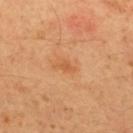The lesion was photographed on a routine skin check and not biopsied; there is no pathology result. Located on the upper back. A lesion tile, about 15 mm wide, cut from a 3D total-body photograph. A male patient approximately 50 years of age.